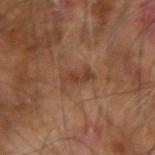– notes: total-body-photography surveillance lesion; no biopsy